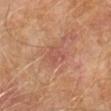Recorded during total-body skin imaging; not selected for excision or biopsy.